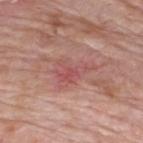The lesion was photographed on a routine skin check and not biopsied; there is no pathology result.
Imaged with white-light lighting.
An algorithmic analysis of the crop reported a lesion area of about 10 mm², an eccentricity of roughly 0.8, and a shape-asymmetry score of about 0.5 (0 = symmetric). It also reported a mean CIELAB color near L≈53 a*≈27 b*≈23, a lesion–skin lightness drop of about 8, and a normalized lesion–skin contrast near 5.5. It also reported a border-irregularity rating of about 5.5/10, a within-lesion color-variation index near 5/10, and a peripheral color-asymmetry measure near 1.5. And it measured a nevus-likeness score of about 0/100.
The patient is a male in their 60s.
Located on the upper back.
A region of skin cropped from a whole-body photographic capture, roughly 15 mm wide.